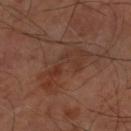Clinical impression:
Recorded during total-body skin imaging; not selected for excision or biopsy.
Image and clinical context:
A patient roughly 65 years of age. Cropped from a total-body skin-imaging series; the visible field is about 15 mm. The tile uses cross-polarized illumination. The lesion-visualizer software estimated a border-irregularity rating of about 9.5/10, a within-lesion color-variation index near 4.5/10, and a peripheral color-asymmetry measure near 1.5. It also reported an automated nevus-likeness rating near 0 out of 100 and a lesion-detection confidence of about 100/100. Located on the right forearm. The recorded lesion diameter is about 6.5 mm.